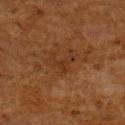  biopsy_status: not biopsied; imaged during a skin examination
  site: upper back
  patient:
    sex: female
    age_approx: 50
  image:
    source: total-body photography crop
    field_of_view_mm: 15
  lighting: cross-polarized
  automated_metrics:
    area_mm2_approx: 5.0
    eccentricity: 0.65
    shape_asymmetry: 0.75
    cielab_L: 27
    cielab_a: 18
    cielab_b: 28
    vs_skin_darker_L: 5.0
    vs_skin_contrast_norm: 5.0
    border_irregularity_0_10: 8.5
    peripheral_color_asymmetry: 0.5